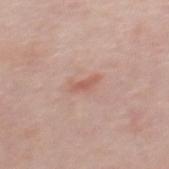Q: Was this lesion biopsied?
A: imaged on a skin check; not biopsied
Q: Who is the patient?
A: male, in their 40s
Q: Lesion location?
A: the mid back
Q: What is the imaging modality?
A: 15 mm crop, total-body photography